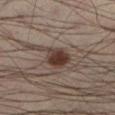biopsy status: total-body-photography surveillance lesion; no biopsy
anatomic site: the leg
tile lighting: cross-polarized
automated lesion analysis: a shape eccentricity near 0.4 and a shape-asymmetry score of about 0.2 (0 = symmetric); an average lesion color of about L≈36 a*≈15 b*≈22 (CIELAB) and a normalized lesion–skin contrast near 11; border irregularity of about 2 on a 0–10 scale, a within-lesion color-variation index near 4.5/10, and radial color variation of about 1.5
imaging modality: 15 mm crop, total-body photography
size: ~3.5 mm (longest diameter)
patient: male, approximately 40 years of age